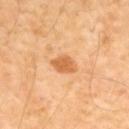Image and clinical context: An algorithmic analysis of the crop reported a lesion area of about 5 mm² and an outline eccentricity of about 0.75 (0 = round, 1 = elongated). The analysis additionally found border irregularity of about 2.5 on a 0–10 scale, a within-lesion color-variation index near 1.5/10, and a peripheral color-asymmetry measure near 0.5. A 15 mm close-up extracted from a 3D total-body photography capture. Captured under cross-polarized illumination. The recorded lesion diameter is about 3 mm. On the mid back. A male subject, aged 53–57.